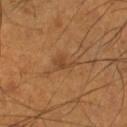follow-up: imaged on a skin check; not biopsied
tile lighting: cross-polarized
site: the right lower leg
TBP lesion metrics: an area of roughly 3 mm², an outline eccentricity of about 0.8 (0 = round, 1 = elongated), and a symmetry-axis asymmetry near 0.5; border irregularity of about 5 on a 0–10 scale, a within-lesion color-variation index near 1.5/10, and a peripheral color-asymmetry measure near 0.5; a classifier nevus-likeness of about 0/100 and a detector confidence of about 100 out of 100 that the crop contains a lesion
acquisition: ~15 mm crop, total-body skin-cancer survey
subject: male, aged 53 to 57